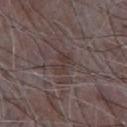This lesion was catalogued during total-body skin photography and was not selected for biopsy.
A male subject, aged approximately 65.
Automated tile analysis of the lesion measured a mean CIELAB color near L≈35 a*≈14 b*≈17, a lesion–skin lightness drop of about 6, and a normalized border contrast of about 6. The analysis additionally found a border-irregularity rating of about 4/10, a within-lesion color-variation index near 0/10, and peripheral color asymmetry of about 0.
This is a white-light tile.
Located on the front of the torso.
A roughly 15 mm field-of-view crop from a total-body skin photograph.